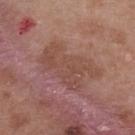Captured during whole-body skin photography for melanoma surveillance; the lesion was not biopsied. Cropped from a whole-body photographic skin survey; the tile spans about 15 mm. Imaged with white-light lighting. The subject is a female aged 38 to 42. Located on the upper back. Automated image analysis of the tile measured a border-irregularity index near 6.5/10, a color-variation rating of about 3.5/10, and a peripheral color-asymmetry measure near 1. About 6.5 mm across.